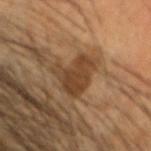Q: Is there a histopathology result?
A: no biopsy performed (imaged during a skin exam)
Q: Where on the body is the lesion?
A: the head or neck
Q: What are the patient's age and sex?
A: male, aged 53–57
Q: Illumination type?
A: cross-polarized illumination
Q: Automated lesion metrics?
A: a lesion area of about 13 mm² and an outline eccentricity of about 0.3 (0 = round, 1 = elongated); a lesion color around L≈38 a*≈17 b*≈30 in CIELAB, about 9 CIELAB-L* units darker than the surrounding skin, and a lesion-to-skin contrast of about 7.5 (normalized; higher = more distinct); a border-irregularity index near 5/10, a color-variation rating of about 3/10, and radial color variation of about 1
Q: How was this image acquired?
A: total-body-photography crop, ~15 mm field of view
Q: What is the lesion's diameter?
A: ~4.5 mm (longest diameter)